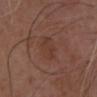Clinical impression: Imaged during a routine full-body skin examination; the lesion was not biopsied and no histopathology is available. Acquisition and patient details: Captured under white-light illumination. The recorded lesion diameter is about 3.5 mm. The lesion is located on the chest. A 15 mm close-up extracted from a 3D total-body photography capture. The subject is a male aged 73–77.